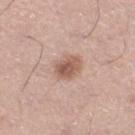Assessment:
The lesion was photographed on a routine skin check and not biopsied; there is no pathology result.
Clinical summary:
The tile uses white-light illumination. Measured at roughly 3.5 mm in maximum diameter. A male patient in their mid-30s. The lesion is on the left lower leg. An algorithmic analysis of the crop reported a lesion–skin lightness drop of about 12. A close-up tile cropped from a whole-body skin photograph, about 15 mm across.